Located on the left thigh; a female subject, aged around 55; a lesion tile, about 15 mm wide, cut from a 3D total-body photograph:
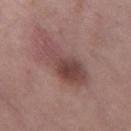Background:
Automated tile analysis of the lesion measured internal color variation of about 6 on a 0–10 scale and a peripheral color-asymmetry measure near 2. The recorded lesion diameter is about 7.5 mm. Captured under white-light illumination.
Diagnosis:
The lesion was biopsied, and histopathology showed a dysplastic (Clark) nevus.